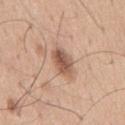Q: Who is the patient?
A: male, in their mid-60s
Q: What is the imaging modality?
A: total-body-photography crop, ~15 mm field of view
Q: Lesion location?
A: the mid back